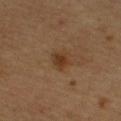Assessment: The lesion was tiled from a total-body skin photograph and was not biopsied. Acquisition and patient details: Captured under cross-polarized illumination. A male patient, in their mid-50s. Measured at roughly 2.5 mm in maximum diameter. A 15 mm close-up tile from a total-body photography series done for melanoma screening. Located on the arm.